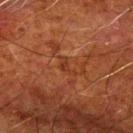Q: Is there a histopathology result?
A: catalogued during a skin exam; not biopsied
Q: What is the anatomic site?
A: the left upper arm
Q: Patient demographics?
A: male, approximately 80 years of age
Q: How was the tile lit?
A: cross-polarized
Q: How was this image acquired?
A: 15 mm crop, total-body photography
Q: Automated lesion metrics?
A: a shape eccentricity near 0.9 and two-axis asymmetry of about 0.55; an average lesion color of about L≈29 a*≈22 b*≈29 (CIELAB), a lesion–skin lightness drop of about 5, and a normalized border contrast of about 5.5; border irregularity of about 5.5 on a 0–10 scale; a detector confidence of about 85 out of 100 that the crop contains a lesion